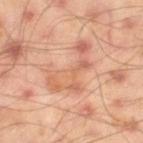Assessment:
Imaged during a routine full-body skin examination; the lesion was not biopsied and no histopathology is available.
Image and clinical context:
A male patient, aged 43 to 47. A close-up tile cropped from a whole-body skin photograph, about 15 mm across. Captured under cross-polarized illumination. The lesion is located on the right thigh. The recorded lesion diameter is about 6.5 mm.